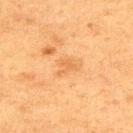The lesion was photographed on a routine skin check and not biopsied; there is no pathology result.
The lesion-visualizer software estimated an eccentricity of roughly 0.65. The software also gave a border-irregularity index near 5.5/10, a color-variation rating of about 0.5/10, and radial color variation of about 0.
The lesion is located on the upper back.
Captured under cross-polarized illumination.
The recorded lesion diameter is about 2.5 mm.
The patient is a male in their mid- to late 70s.
Cropped from a whole-body photographic skin survey; the tile spans about 15 mm.